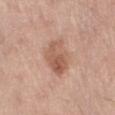Q: Was a biopsy performed?
A: imaged on a skin check; not biopsied
Q: What is the anatomic site?
A: the left thigh
Q: How was this image acquired?
A: total-body-photography crop, ~15 mm field of view
Q: What is the lesion's diameter?
A: ~4.5 mm (longest diameter)
Q: Patient demographics?
A: male, aged approximately 70